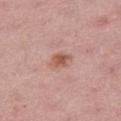<tbp_lesion>
<biopsy_status>not biopsied; imaged during a skin examination</biopsy_status>
<site>right thigh</site>
<patient>
  <sex>female</sex>
  <age_approx>45</age_approx>
</patient>
<image>
  <source>total-body photography crop</source>
  <field_of_view_mm>15</field_of_view_mm>
</image>
<lesion_size>
  <long_diameter_mm_approx>3.0</long_diameter_mm_approx>
</lesion_size>
<lighting>white-light</lighting>
</tbp_lesion>